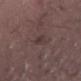{"biopsy_status": "not biopsied; imaged during a skin examination", "lighting": "white-light", "lesion_size": {"long_diameter_mm_approx": 2.5}, "site": "right thigh", "patient": {"sex": "male", "age_approx": 50}, "image": {"source": "total-body photography crop", "field_of_view_mm": 15}}